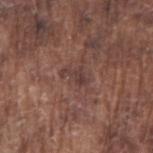Part of a total-body skin-imaging series; this lesion was reviewed on a skin check and was not flagged for biopsy. The patient is a male approximately 75 years of age. This is a white-light tile. The lesion is located on the right upper arm. The lesion's longest dimension is about 3 mm. Cropped from a whole-body photographic skin survey; the tile spans about 15 mm.